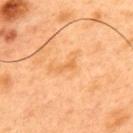Clinical impression:
Captured during whole-body skin photography for melanoma surveillance; the lesion was not biopsied.
Acquisition and patient details:
A roughly 15 mm field-of-view crop from a total-body skin photograph. The recorded lesion diameter is about 2.5 mm. A male subject, approximately 50 years of age. The lesion is on the upper back. Imaged with cross-polarized lighting.